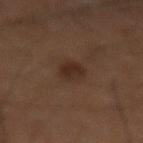notes=catalogued during a skin exam; not biopsied
tile lighting=cross-polarized illumination
patient=male, aged 58 to 62
image source=~15 mm crop, total-body skin-cancer survey
automated metrics=a border-irregularity rating of about 1.5/10 and a peripheral color-asymmetry measure near 1
body site=the mid back